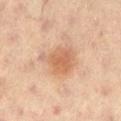No biopsy was performed on this lesion — it was imaged during a full skin examination and was not determined to be concerning. A region of skin cropped from a whole-body photographic capture, roughly 15 mm wide. Captured under cross-polarized illumination. The lesion is located on the left thigh. An algorithmic analysis of the crop reported a mean CIELAB color near L≈58 a*≈20 b*≈33, about 10 CIELAB-L* units darker than the surrounding skin, and a lesion-to-skin contrast of about 6.5 (normalized; higher = more distinct). The software also gave a border-irregularity index near 4/10 and radial color variation of about 1. A male patient, in their 60s.